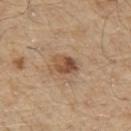{
  "patient": {
    "sex": "male",
    "age_approx": 70
  },
  "image": {
    "source": "total-body photography crop",
    "field_of_view_mm": 15
  },
  "site": "chest"
}